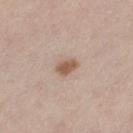workup: total-body-photography surveillance lesion; no biopsy | diameter: about 3 mm | body site: the left thigh | imaging modality: total-body-photography crop, ~15 mm field of view | subject: male, about 60 years old.